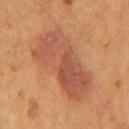Clinical summary:
Cropped from a total-body skin-imaging series; the visible field is about 15 mm. A male patient, in their mid- to late 50s. Located on the mid back. Captured under cross-polarized illumination.